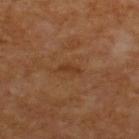* workup — total-body-photography surveillance lesion; no biopsy
* patient — male, aged approximately 60
* imaging modality — ~15 mm crop, total-body skin-cancer survey
* tile lighting — cross-polarized illumination
* body site — the back
* image-analysis metrics — a footprint of about 2.5 mm², an eccentricity of roughly 0.9, and two-axis asymmetry of about 0.35; a lesion-to-skin contrast of about 6 (normalized; higher = more distinct); a border-irregularity rating of about 3.5/10, a color-variation rating of about 0/10, and radial color variation of about 0